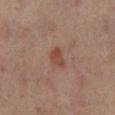Clinical impression: Captured during whole-body skin photography for melanoma surveillance; the lesion was not biopsied. Context: A female subject aged approximately 50. The lesion is on the left lower leg. This is a cross-polarized tile. The lesion-visualizer software estimated a footprint of about 3.5 mm². The analysis additionally found an automated nevus-likeness rating near 45 out of 100 and a lesion-detection confidence of about 100/100. A lesion tile, about 15 mm wide, cut from a 3D total-body photograph.